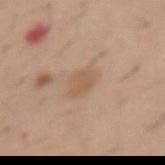The lesion was tiled from a total-body skin photograph and was not biopsied.
The lesion is on the abdomen.
Captured under white-light illumination.
The total-body-photography lesion software estimated an area of roughly 6 mm², an eccentricity of roughly 0.7, and a symmetry-axis asymmetry near 0.25. The software also gave an average lesion color of about L≈57 a*≈17 b*≈31 (CIELAB), about 7 CIELAB-L* units darker than the surrounding skin, and a lesion-to-skin contrast of about 5 (normalized; higher = more distinct). And it measured a border-irregularity rating of about 2.5/10, a within-lesion color-variation index near 1.5/10, and a peripheral color-asymmetry measure near 0.5.
Longest diameter approximately 3 mm.
A male subject aged around 60.
A lesion tile, about 15 mm wide, cut from a 3D total-body photograph.